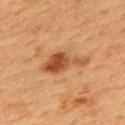| feature | finding |
|---|---|
| site | the upper back |
| size | about 6 mm |
| automated metrics | an average lesion color of about L≈41 a*≈21 b*≈33 (CIELAB), about 11 CIELAB-L* units darker than the surrounding skin, and a normalized border contrast of about 8.5 |
| lighting | cross-polarized illumination |
| imaging modality | ~15 mm crop, total-body skin-cancer survey |
| subject | female, aged 53–57 |